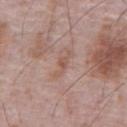biopsy status=total-body-photography surveillance lesion; no biopsy | tile lighting=white-light illumination | automated lesion analysis=a border-irregularity rating of about 6.5/10, internal color variation of about 1.5 on a 0–10 scale, and peripheral color asymmetry of about 0.5 | diameter=≈3.5 mm | imaging modality=~15 mm tile from a whole-body skin photo | body site=the abdomen | subject=male, aged around 70.